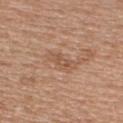* biopsy status · total-body-photography surveillance lesion; no biopsy
* image · total-body-photography crop, ~15 mm field of view
* location · the arm
* lighting · white-light
* patient · female, aged 38–42
* lesion diameter · ~3.5 mm (longest diameter)